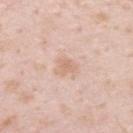Case summary:
- biopsy status — imaged on a skin check; not biopsied
- diameter — ~2.5 mm (longest diameter)
- image — ~15 mm tile from a whole-body skin photo
- location — the upper back
- patient — male, aged 33 to 37
- automated lesion analysis — a border-irregularity rating of about 4/10, internal color variation of about 1.5 on a 0–10 scale, and radial color variation of about 0.5; a nevus-likeness score of about 0/100
- lighting — white-light